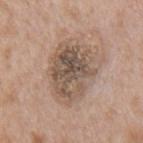notes = imaged on a skin check; not biopsied
diameter = ≈6 mm
imaging modality = ~15 mm crop, total-body skin-cancer survey
image-analysis metrics = a lesion color around L≈53 a*≈14 b*≈26 in CIELAB, a lesion–skin lightness drop of about 11, and a normalized border contrast of about 8; border irregularity of about 3 on a 0–10 scale, a within-lesion color-variation index near 7.5/10, and a peripheral color-asymmetry measure near 2.5
patient = male, in their mid-60s
anatomic site = the mid back